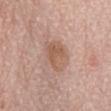{
  "biopsy_status": "not biopsied; imaged during a skin examination",
  "lighting": "white-light",
  "lesion_size": {
    "long_diameter_mm_approx": 4.5
  },
  "image": {
    "source": "total-body photography crop",
    "field_of_view_mm": 15
  },
  "site": "chest",
  "automated_metrics": {
    "area_mm2_approx": 11.0,
    "eccentricity": 0.7
  },
  "patient": {
    "sex": "female",
    "age_approx": 65
  }
}